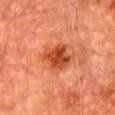| feature | finding |
|---|---|
| biopsy status | imaged on a skin check; not biopsied |
| body site | the chest |
| TBP lesion metrics | an outline eccentricity of about 0.45 (0 = round, 1 = elongated) and a symmetry-axis asymmetry near 0.2; a lesion color around L≈44 a*≈32 b*≈38 in CIELAB, about 12 CIELAB-L* units darker than the surrounding skin, and a lesion-to-skin contrast of about 9 (normalized; higher = more distinct) |
| subject | male, aged approximately 65 |
| lighting | cross-polarized illumination |
| imaging modality | ~15 mm tile from a whole-body skin photo |